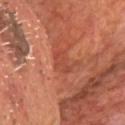Notes:
• diameter — ≈3 mm
• body site — the chest
• patient — male, about 70 years old
• lighting — cross-polarized
• acquisition — ~15 mm crop, total-body skin-cancer survey